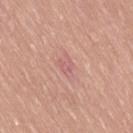Impression:
Recorded during total-body skin imaging; not selected for excision or biopsy.
Acquisition and patient details:
The lesion is on the lower back. This image is a 15 mm lesion crop taken from a total-body photograph. Captured under white-light illumination. The patient is a male approximately 60 years of age. The total-body-photography lesion software estimated a border-irregularity rating of about 3/10 and a within-lesion color-variation index near 1/10. It also reported an automated nevus-likeness rating near 0 out of 100.